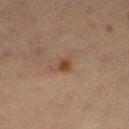workup: total-body-photography surveillance lesion; no biopsy
site: the left thigh
subject: female, approximately 30 years of age
image: ~15 mm tile from a whole-body skin photo